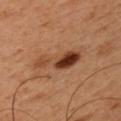Part of a total-body skin-imaging series; this lesion was reviewed on a skin check and was not flagged for biopsy.
The patient is a male aged 48–52.
Located on the left upper arm.
A 15 mm close-up extracted from a 3D total-body photography capture.